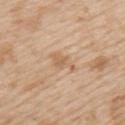The lesion was tiled from a total-body skin photograph and was not biopsied.
A male patient, in their mid- to late 60s.
The lesion is on the upper back.
Automated tile analysis of the lesion measured a lesion area of about 6 mm², an outline eccentricity of about 0.85 (0 = round, 1 = elongated), and a symmetry-axis asymmetry near 0.35. And it measured an average lesion color of about L≈63 a*≈17 b*≈34 (CIELAB), about 7 CIELAB-L* units darker than the surrounding skin, and a lesion-to-skin contrast of about 5 (normalized; higher = more distinct). And it measured a border-irregularity index near 4/10. It also reported a nevus-likeness score of about 0/100.
A 15 mm crop from a total-body photograph taken for skin-cancer surveillance.
The lesion's longest dimension is about 4 mm.
Captured under white-light illumination.